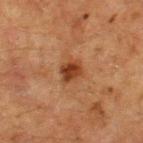- workup · catalogued during a skin exam; not biopsied
- size · about 2.5 mm
- site · the left thigh
- automated metrics · a footprint of about 4.5 mm², an eccentricity of roughly 0.6, and a shape-asymmetry score of about 0.3 (0 = symmetric); a lesion color around L≈33 a*≈22 b*≈31 in CIELAB and about 11 CIELAB-L* units darker than the surrounding skin; a lesion-detection confidence of about 100/100
- illumination · cross-polarized illumination
- patient · female, about 50 years old
- image source · ~15 mm crop, total-body skin-cancer survey